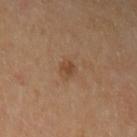Notes:
- biopsy status: imaged on a skin check; not biopsied
- imaging modality: ~15 mm tile from a whole-body skin photo
- tile lighting: cross-polarized illumination
- automated lesion analysis: a mean CIELAB color near L≈45 a*≈20 b*≈33, roughly 8 lightness units darker than nearby skin, and a lesion-to-skin contrast of about 7 (normalized; higher = more distinct); a classifier nevus-likeness of about 10/100
- subject: female, in their mid-50s
- anatomic site: the left upper arm
- diameter: ~1.5 mm (longest diameter)